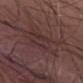workup: no biopsy performed (imaged during a skin exam)
patient: male, in their mid-70s
illumination: white-light illumination
site: the abdomen
automated lesion analysis: a lesion area of about 18 mm², an eccentricity of roughly 0.7, and a shape-asymmetry score of about 0.3 (0 = symmetric); a border-irregularity rating of about 4.5/10; an automated nevus-likeness rating near 0 out of 100 and lesion-presence confidence of about 55/100
size: about 6 mm
image source: 15 mm crop, total-body photography